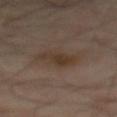Captured during whole-body skin photography for melanoma surveillance; the lesion was not biopsied. The subject is a male aged approximately 50. The lesion is located on the back. An algorithmic analysis of the crop reported a mean CIELAB color near L≈34 a*≈13 b*≈24, about 7 CIELAB-L* units darker than the surrounding skin, and a normalized border contrast of about 7.5. The analysis additionally found a border-irregularity index near 3.5/10, a within-lesion color-variation index near 3/10, and a peripheral color-asymmetry measure near 1. And it measured an automated nevus-likeness rating near 30 out of 100 and a lesion-detection confidence of about 100/100. Measured at roughly 3.5 mm in maximum diameter. A 15 mm crop from a total-body photograph taken for skin-cancer surveillance. The tile uses cross-polarized illumination.